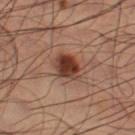Case summary:
– workup: imaged on a skin check; not biopsied